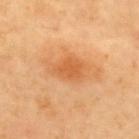<tbp_lesion>
  <biopsy_status>not biopsied; imaged during a skin examination</biopsy_status>
  <patient>
    <sex>male</sex>
    <age_approx>55</age_approx>
  </patient>
  <lighting>cross-polarized</lighting>
  <image>
    <source>total-body photography crop</source>
    <field_of_view_mm>15</field_of_view_mm>
  </image>
  <site>upper back</site>
  <automated_metrics>
    <area_mm2_approx>6.0</area_mm2_approx>
    <eccentricity>0.6</eccentricity>
    <shape_asymmetry>0.25</shape_asymmetry>
    <cielab_L>60</cielab_L>
    <cielab_a>29</cielab_a>
    <cielab_b>46</cielab_b>
    <vs_skin_darker_L>9.0</vs_skin_darker_L>
    <vs_skin_contrast_norm>6.5</vs_skin_contrast_norm>
    <border_irregularity_0_10>2.5</border_irregularity_0_10>
  </automated_metrics>
</tbp_lesion>